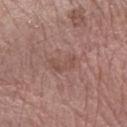notes = total-body-photography surveillance lesion; no biopsy
lesion diameter = ≈3.5 mm
subject = male, aged 53 to 57
imaging modality = total-body-photography crop, ~15 mm field of view
TBP lesion metrics = an area of roughly 5 mm², a shape eccentricity near 0.85, and two-axis asymmetry of about 0.5
body site = the left forearm